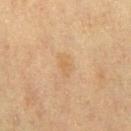Clinical impression:
Recorded during total-body skin imaging; not selected for excision or biopsy.
Acquisition and patient details:
An algorithmic analysis of the crop reported a lesion area of about 4 mm², an eccentricity of roughly 0.75, and a symmetry-axis asymmetry near 0.3. It also reported an average lesion color of about L≈54 a*≈15 b*≈34 (CIELAB), about 5 CIELAB-L* units darker than the surrounding skin, and a normalized lesion–skin contrast near 4.5. The software also gave border irregularity of about 3.5 on a 0–10 scale, a within-lesion color-variation index near 2/10, and peripheral color asymmetry of about 0.5. And it measured a classifier nevus-likeness of about 0/100. The lesion is on the right thigh. The subject is a female in their 40s. Approximately 3 mm at its widest. Captured under cross-polarized illumination. A roughly 15 mm field-of-view crop from a total-body skin photograph.